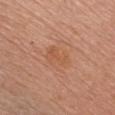Clinical impression: The lesion was photographed on a routine skin check and not biopsied; there is no pathology result. Clinical summary: A female subject aged approximately 60. A lesion tile, about 15 mm wide, cut from a 3D total-body photograph. The lesion's longest dimension is about 4 mm. From the chest. Automated tile analysis of the lesion measured a lesion color around L≈55 a*≈24 b*≈34 in CIELAB, roughly 5 lightness units darker than nearby skin, and a normalized border contrast of about 5. The tile uses white-light illumination.